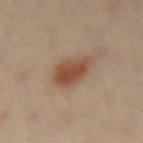{
  "biopsy_status": "not biopsied; imaged during a skin examination",
  "lesion_size": {
    "long_diameter_mm_approx": 3.5
  },
  "site": "lower back",
  "lighting": "cross-polarized",
  "image": {
    "source": "total-body photography crop",
    "field_of_view_mm": 15
  },
  "automated_metrics": {
    "area_mm2_approx": 9.5,
    "eccentricity": 0.55,
    "shape_asymmetry": 0.25,
    "nevus_likeness_0_100": 100
  },
  "patient": {
    "sex": "male",
    "age_approx": 55
  }
}